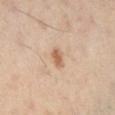– follow-up · imaged on a skin check; not biopsied
– size · ~2 mm (longest diameter)
– image-analysis metrics · a color-variation rating of about 2/10 and a peripheral color-asymmetry measure near 0.5; a nevus-likeness score of about 85/100 and a lesion-detection confidence of about 100/100
– anatomic site · the right lower leg
– subject · female, aged approximately 40
– imaging modality · ~15 mm crop, total-body skin-cancer survey